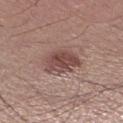Clinical impression: Captured during whole-body skin photography for melanoma surveillance; the lesion was not biopsied. Clinical summary: Cropped from a whole-body photographic skin survey; the tile spans about 15 mm. From the right lower leg. The patient is a male in their mid-50s.